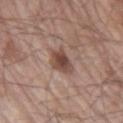| field | value |
|---|---|
| workup | no biopsy performed (imaged during a skin exam) |
| imaging modality | ~15 mm tile from a whole-body skin photo |
| TBP lesion metrics | a footprint of about 6.5 mm², an eccentricity of roughly 0.35, and a shape-asymmetry score of about 0.3 (0 = symmetric); an average lesion color of about L≈46 a*≈19 b*≈24 (CIELAB), roughly 12 lightness units darker than nearby skin, and a lesion-to-skin contrast of about 9 (normalized; higher = more distinct); a border-irregularity rating of about 3/10 and radial color variation of about 1; an automated nevus-likeness rating near 90 out of 100 and a lesion-detection confidence of about 100/100 |
| body site | the right upper arm |
| lesion diameter | ≈3 mm |
| lighting | white-light |
| patient | male, in their mid- to late 60s |